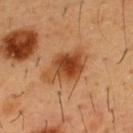A male subject, aged approximately 55.
Approximately 5.5 mm at its widest.
The lesion is located on the chest.
A roughly 15 mm field-of-view crop from a total-body skin photograph.